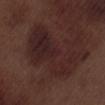Q: Was this lesion biopsied?
A: total-body-photography surveillance lesion; no biopsy
Q: Lesion size?
A: about 9 mm
Q: Where on the body is the lesion?
A: the leg
Q: How was this image acquired?
A: ~15 mm tile from a whole-body skin photo
Q: What are the patient's age and sex?
A: male, approximately 70 years of age
Q: Illumination type?
A: white-light illumination